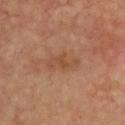Q: Was a biopsy performed?
A: total-body-photography surveillance lesion; no biopsy
Q: What did automated image analysis measure?
A: an eccentricity of roughly 0.9 and a symmetry-axis asymmetry near 0.25; a mean CIELAB color near L≈47 a*≈21 b*≈32 and a normalized border contrast of about 5.5; a border-irregularity index near 3.5/10, a within-lesion color-variation index near 3.5/10, and a peripheral color-asymmetry measure near 1.5
Q: Lesion location?
A: the chest
Q: Patient demographics?
A: male, roughly 65 years of age
Q: How was this image acquired?
A: total-body-photography crop, ~15 mm field of view
Q: How large is the lesion?
A: ~4 mm (longest diameter)
Q: How was the tile lit?
A: cross-polarized illumination